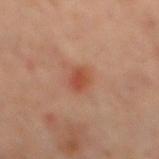Assessment: Recorded during total-body skin imaging; not selected for excision or biopsy. Background: The patient is a male roughly 60 years of age. On the mid back. Imaged with cross-polarized lighting. This image is a 15 mm lesion crop taken from a total-body photograph. Measured at roughly 3 mm in maximum diameter.